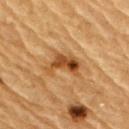  biopsy_status: not biopsied; imaged during a skin examination
  lesion_size:
    long_diameter_mm_approx: 4.5
  image:
    source: total-body photography crop
    field_of_view_mm: 15
  patient:
    sex: male
    age_approx: 85+
  site: right upper arm
  automated_metrics:
    area_mm2_approx: 7.5
    eccentricity: 0.8
    border_irregularity_0_10: 6.0
    peripheral_color_asymmetry: 2.5
    nevus_likeness_0_100: 80
    lesion_detection_confidence_0_100: 100
  lighting: cross-polarized